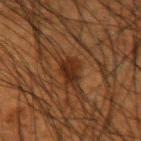notes: catalogued during a skin exam; not biopsied
automated lesion analysis: a lesion area of about 4.5 mm², a shape eccentricity near 0.85, and a shape-asymmetry score of about 0.35 (0 = symmetric); border irregularity of about 3.5 on a 0–10 scale, internal color variation of about 3 on a 0–10 scale, and a peripheral color-asymmetry measure near 1
lesion diameter: ~3 mm (longest diameter)
image: ~15 mm crop, total-body skin-cancer survey
location: the right forearm
lighting: cross-polarized illumination
patient: male, aged 48 to 52